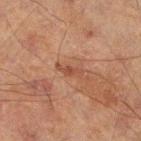Recorded during total-body skin imaging; not selected for excision or biopsy.
The recorded lesion diameter is about 3 mm.
Automated tile analysis of the lesion measured an area of roughly 3 mm², a shape eccentricity near 0.95, and two-axis asymmetry of about 0.35. And it measured an average lesion color of about L≈36 a*≈19 b*≈25 (CIELAB), roughly 7 lightness units darker than nearby skin, and a lesion-to-skin contrast of about 6 (normalized; higher = more distinct).
Captured under cross-polarized illumination.
On the left leg.
A male patient about 60 years old.
A roughly 15 mm field-of-view crop from a total-body skin photograph.